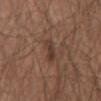The lesion was tiled from a total-body skin photograph and was not biopsied. Measured at roughly 3 mm in maximum diameter. A male patient aged around 65. From the abdomen. A roughly 15 mm field-of-view crop from a total-body skin photograph.